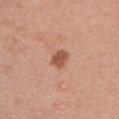Q: Is there a histopathology result?
A: no biopsy performed (imaged during a skin exam)
Q: Where on the body is the lesion?
A: the left upper arm
Q: How was the tile lit?
A: white-light
Q: How was this image acquired?
A: ~15 mm tile from a whole-body skin photo
Q: What did automated image analysis measure?
A: a lesion color around L≈54 a*≈24 b*≈32 in CIELAB, a lesion–skin lightness drop of about 12, and a normalized border contrast of about 8.5
Q: Patient demographics?
A: male, approximately 40 years of age